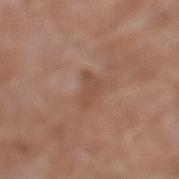biopsy_status: not biopsied; imaged during a skin examination
automated_metrics:
  area_mm2_approx: 4.5
  eccentricity: 0.75
  shape_asymmetry: 0.45
  lesion_detection_confidence_0_100: 100
lighting: white-light
site: left lower leg
patient:
  sex: male
  age_approx: 50
image:
  source: total-body photography crop
  field_of_view_mm: 15
lesion_size:
  long_diameter_mm_approx: 3.0